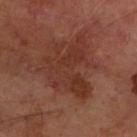Clinical impression:
No biopsy was performed on this lesion — it was imaged during a full skin examination and was not determined to be concerning.
Clinical summary:
Automated tile analysis of the lesion measured a border-irregularity index near 9/10, a color-variation rating of about 3.5/10, and a peripheral color-asymmetry measure near 1. Captured under cross-polarized illumination. A male patient, aged approximately 70. On the left forearm. Cropped from a total-body skin-imaging series; the visible field is about 15 mm.